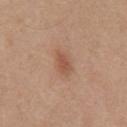Q: Was this lesion biopsied?
A: imaged on a skin check; not biopsied
Q: Who is the patient?
A: male, approximately 70 years of age
Q: How was this image acquired?
A: 15 mm crop, total-body photography
Q: Lesion location?
A: the chest